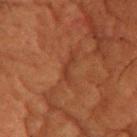Imaged during a routine full-body skin examination; the lesion was not biopsied and no histopathology is available. Imaged with cross-polarized lighting. Cropped from a total-body skin-imaging series; the visible field is about 15 mm. Located on the head or neck. Measured at roughly 3 mm in maximum diameter. The patient is a female roughly 80 years of age.